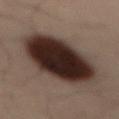No biopsy was performed on this lesion — it was imaged during a full skin examination and was not determined to be concerning.
A lesion tile, about 15 mm wide, cut from a 3D total-body photograph.
Measured at roughly 9 mm in maximum diameter.
Imaged with cross-polarized lighting.
A male patient in their 50s.
Located on the mid back.